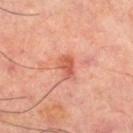Impression: No biopsy was performed on this lesion — it was imaged during a full skin examination and was not determined to be concerning. Image and clinical context: Measured at roughly 3 mm in maximum diameter. On the right thigh. A close-up tile cropped from a whole-body skin photograph, about 15 mm across. An algorithmic analysis of the crop reported an outline eccentricity of about 0.85 (0 = round, 1 = elongated) and a shape-asymmetry score of about 0.25 (0 = symmetric). The software also gave a mean CIELAB color near L≈58 a*≈32 b*≈35, a lesion–skin lightness drop of about 11, and a lesion-to-skin contrast of about 7 (normalized; higher = more distinct). It also reported a lesion-detection confidence of about 100/100. A male subject about 65 years old.